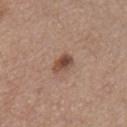Clinical impression: This lesion was catalogued during total-body skin photography and was not selected for biopsy. Clinical summary: Longest diameter approximately 3 mm. A male patient, aged 53–57. Automated image analysis of the tile measured an area of roughly 4.5 mm², an eccentricity of roughly 0.75, and a symmetry-axis asymmetry near 0.25. The software also gave a lesion color around L≈47 a*≈19 b*≈27 in CIELAB, a lesion–skin lightness drop of about 12, and a normalized lesion–skin contrast near 9. The analysis additionally found a nevus-likeness score of about 75/100 and a lesion-detection confidence of about 100/100. Located on the arm. This image is a 15 mm lesion crop taken from a total-body photograph. Imaged with white-light lighting.